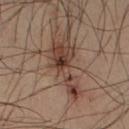Part of a total-body skin-imaging series; this lesion was reviewed on a skin check and was not flagged for biopsy. On the right upper arm. A male patient, aged around 40. The recorded lesion diameter is about 7 mm. A lesion tile, about 15 mm wide, cut from a 3D total-body photograph.